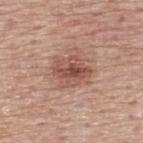lesion_size:
  long_diameter_mm_approx: 4.5
automated_metrics:
  area_mm2_approx: 12.0
  eccentricity: 0.55
  shape_asymmetry: 0.2
  vs_skin_darker_L: 11.0
  vs_skin_contrast_norm: 7.5
image:
  source: total-body photography crop
  field_of_view_mm: 15
site: upper back
patient:
  sex: male
  age_approx: 75
lighting: white-light